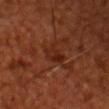Notes:
– location: the head or neck
– tile lighting: cross-polarized illumination
– size: ≈3 mm
– acquisition: ~15 mm tile from a whole-body skin photo
– subject: male, approximately 50 years of age